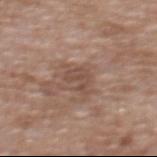Q: Was a biopsy performed?
A: total-body-photography surveillance lesion; no biopsy
Q: Automated lesion metrics?
A: a lesion area of about 4.5 mm², an outline eccentricity of about 0.85 (0 = round, 1 = elongated), and a symmetry-axis asymmetry near 0.55; an average lesion color of about L≈48 a*≈18 b*≈25 (CIELAB), roughly 7 lightness units darker than nearby skin, and a lesion-to-skin contrast of about 5.5 (normalized; higher = more distinct); a detector confidence of about 100 out of 100 that the crop contains a lesion
Q: Lesion location?
A: the chest
Q: What kind of image is this?
A: ~15 mm crop, total-body skin-cancer survey
Q: How was the tile lit?
A: white-light illumination
Q: Who is the patient?
A: male, aged around 60